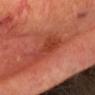{"patient": {"sex": "male", "age_approx": 65}, "image": {"source": "total-body photography crop", "field_of_view_mm": 15}, "lesion_size": {"long_diameter_mm_approx": 4.5}, "site": "head or neck", "automated_metrics": {"eccentricity": 0.8, "shape_asymmetry": 0.45, "cielab_L": 40, "cielab_a": 32, "cielab_b": 33, "vs_skin_darker_L": 8.0, "vs_skin_contrast_norm": 6.0, "nevus_likeness_0_100": 10, "lesion_detection_confidence_0_100": 100}}